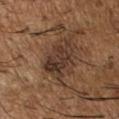Part of a total-body skin-imaging series; this lesion was reviewed on a skin check and was not flagged for biopsy.
The lesion is located on the right forearm.
A 15 mm close-up extracted from a 3D total-body photography capture.
A male subject, roughly 65 years of age.